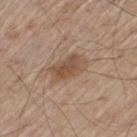Acquisition and patient details: A male subject, in their 60s. The lesion is located on the left thigh. Automated image analysis of the tile measured a symmetry-axis asymmetry near 0.25. A roughly 15 mm field-of-view crop from a total-body skin photograph. Captured under white-light illumination.